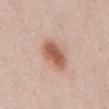lighting: white-light
TBP lesion metrics: a footprint of about 11 mm², a shape eccentricity near 0.7, and a shape-asymmetry score of about 0.2 (0 = symmetric); roughly 13 lightness units darker than nearby skin and a lesion-to-skin contrast of about 8.5 (normalized; higher = more distinct); an automated nevus-likeness rating near 100 out of 100 and lesion-presence confidence of about 100/100
subject: female, about 40 years old
imaging modality: total-body-photography crop, ~15 mm field of view
lesion size: ~4 mm (longest diameter)
location: the abdomen